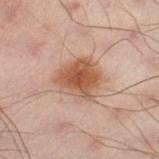Q: Was this lesion biopsied?
A: total-body-photography surveillance lesion; no biopsy
Q: Who is the patient?
A: male, aged approximately 50
Q: Lesion size?
A: ≈4.5 mm
Q: What is the imaging modality?
A: total-body-photography crop, ~15 mm field of view
Q: What is the anatomic site?
A: the left leg